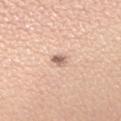Imaged during a routine full-body skin examination; the lesion was not biopsied and no histopathology is available. An algorithmic analysis of the crop reported a footprint of about 5 mm², a shape eccentricity near 0.5, and a shape-asymmetry score of about 0.2 (0 = symmetric). And it measured about 10 CIELAB-L* units darker than the surrounding skin and a normalized lesion–skin contrast near 5.5. The analysis additionally found a border-irregularity rating of about 2/10, internal color variation of about 7 on a 0–10 scale, and radial color variation of about 2.5. It also reported a classifier nevus-likeness of about 65/100 and a detector confidence of about 100 out of 100 that the crop contains a lesion. A female patient, aged approximately 30. Cropped from a whole-body photographic skin survey; the tile spans about 15 mm. This is a white-light tile. On the right upper arm. About 2.5 mm across.